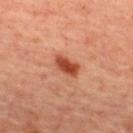Case summary:
* workup: catalogued during a skin exam; not biopsied
* imaging modality: ~15 mm tile from a whole-body skin photo
* size: ≈3.5 mm
* illumination: cross-polarized
* site: the upper back
* subject: female, approximately 45 years of age